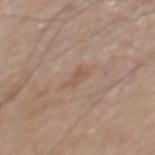| key | value |
|---|---|
| biopsy status | imaged on a skin check; not biopsied |
| body site | the upper back |
| patient | male, approximately 65 years of age |
| automated metrics | a footprint of about 3 mm², a shape eccentricity near 0.85, and a symmetry-axis asymmetry near 0.45; an automated nevus-likeness rating near 0 out of 100 and lesion-presence confidence of about 100/100 |
| lighting | white-light illumination |
| imaging modality | 15 mm crop, total-body photography |
| diameter | about 2.5 mm |